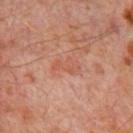Captured during whole-body skin photography for melanoma surveillance; the lesion was not biopsied.
Cropped from a total-body skin-imaging series; the visible field is about 15 mm.
The lesion is on the chest.
The total-body-photography lesion software estimated a lesion color around L≈51 a*≈24 b*≈30 in CIELAB, roughly 5 lightness units darker than nearby skin, and a lesion-to-skin contrast of about 4.5 (normalized; higher = more distinct). It also reported a border-irregularity index near 5.5/10 and peripheral color asymmetry of about 0. It also reported a nevus-likeness score of about 0/100 and a detector confidence of about 100 out of 100 that the crop contains a lesion.
The subject is a male aged 58–62.